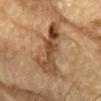Imaged during a routine full-body skin examination; the lesion was not biopsied and no histopathology is available.
On the left forearm.
A close-up tile cropped from a whole-body skin photograph, about 15 mm across.
A male patient aged 83 or over.